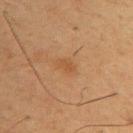notes: catalogued during a skin exam; not biopsied | automated lesion analysis: a mean CIELAB color near L≈45 a*≈19 b*≈34, a lesion–skin lightness drop of about 5, and a lesion-to-skin contrast of about 5 (normalized; higher = more distinct); a lesion-detection confidence of about 100/100 | subject: male, aged approximately 55 | acquisition: 15 mm crop, total-body photography | anatomic site: the chest.